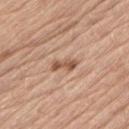{"biopsy_status": "not biopsied; imaged during a skin examination", "site": "left thigh", "lesion_size": {"long_diameter_mm_approx": 3.5}, "lighting": "white-light", "image": {"source": "total-body photography crop", "field_of_view_mm": 15}, "automated_metrics": {"area_mm2_approx": 4.0, "eccentricity": 0.9, "shape_asymmetry": 0.3}, "patient": {"sex": "male", "age_approx": 70}}